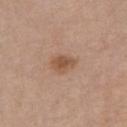| field | value |
|---|---|
| workup | catalogued during a skin exam; not biopsied |
| lighting | white-light |
| image source | ~15 mm crop, total-body skin-cancer survey |
| diameter | about 3 mm |
| body site | the chest |
| subject | male, about 65 years old |
| image-analysis metrics | a classifier nevus-likeness of about 85/100 and a lesion-detection confidence of about 100/100 |